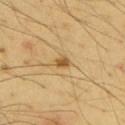biopsy status = total-body-photography surveillance lesion; no biopsy | anatomic site = the upper back | lighting = cross-polarized illumination | patient = male, about 65 years old | acquisition = total-body-photography crop, ~15 mm field of view | automated lesion analysis = a footprint of about 2.5 mm² and an eccentricity of roughly 0.7; a border-irregularity index near 2.5/10, a color-variation rating of about 1/10, and radial color variation of about 0; a nevus-likeness score of about 95/100 and a lesion-detection confidence of about 100/100.